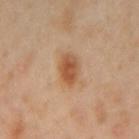notes = total-body-photography surveillance lesion; no biopsy | anatomic site = the left forearm | imaging modality = 15 mm crop, total-body photography | TBP lesion metrics = internal color variation of about 4.5 on a 0–10 scale and peripheral color asymmetry of about 1.5; an automated nevus-likeness rating near 100 out of 100 and lesion-presence confidence of about 100/100 | lesion size = ~3.5 mm (longest diameter) | subject = female, in their 40s | lighting = cross-polarized illumination.